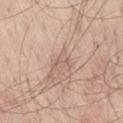No biopsy was performed on this lesion — it was imaged during a full skin examination and was not determined to be concerning. Captured under white-light illumination. This image is a 15 mm lesion crop taken from a total-body photograph. On the right thigh. The total-body-photography lesion software estimated an area of roughly 3 mm², an outline eccentricity of about 0.7 (0 = round, 1 = elongated), and a shape-asymmetry score of about 0.7 (0 = symmetric). The analysis additionally found a lesion color around L≈61 a*≈19 b*≈25 in CIELAB, about 7 CIELAB-L* units darker than the surrounding skin, and a normalized border contrast of about 4.5. The software also gave an automated nevus-likeness rating near 0 out of 100 and a detector confidence of about 60 out of 100 that the crop contains a lesion. The lesion's longest dimension is about 3 mm. A male subject, aged 63–67.